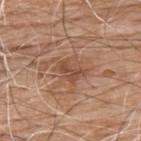The lesion was tiled from a total-body skin photograph and was not biopsied.
A male patient roughly 60 years of age.
About 3.5 mm across.
The tile uses white-light illumination.
Automated image analysis of the tile measured an area of roughly 6 mm² and two-axis asymmetry of about 0.4. The software also gave a lesion color around L≈49 a*≈21 b*≈30 in CIELAB and a normalized lesion–skin contrast near 6.5. The software also gave a nevus-likeness score of about 0/100 and a detector confidence of about 100 out of 100 that the crop contains a lesion.
The lesion is on the upper back.
A roughly 15 mm field-of-view crop from a total-body skin photograph.